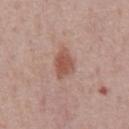Impression: Imaged during a routine full-body skin examination; the lesion was not biopsied and no histopathology is available. Acquisition and patient details: Automated image analysis of the tile measured an average lesion color of about L≈53 a*≈22 b*≈26 (CIELAB) and a normalized border contrast of about 7.5. And it measured a border-irregularity rating of about 2/10, a within-lesion color-variation index near 2.5/10, and radial color variation of about 0.5. A male subject, in their mid- to late 70s. A close-up tile cropped from a whole-body skin photograph, about 15 mm across. This is a white-light tile. The recorded lesion diameter is about 4 mm. On the chest.